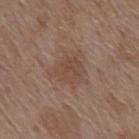{
  "biopsy_status": "not biopsied; imaged during a skin examination",
  "automated_metrics": {
    "area_mm2_approx": 13.0,
    "shape_asymmetry": 0.35,
    "cielab_L": 47,
    "cielab_a": 17,
    "cielab_b": 26,
    "vs_skin_darker_L": 6.0,
    "nevus_likeness_0_100": 0,
    "lesion_detection_confidence_0_100": 100
  },
  "patient": {
    "sex": "female",
    "age_approx": 70
  },
  "site": "upper back",
  "lighting": "white-light",
  "image": {
    "source": "total-body photography crop",
    "field_of_view_mm": 15
  }
}